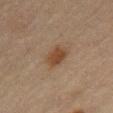This is a cross-polarized tile. The lesion is on the abdomen. The recorded lesion diameter is about 3 mm. A male patient aged around 70. Cropped from a whole-body photographic skin survey; the tile spans about 15 mm.